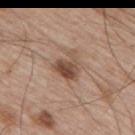Q: How was this image acquired?
A: ~15 mm tile from a whole-body skin photo
Q: What did automated image analysis measure?
A: a lesion area of about 7.5 mm² and a shape-asymmetry score of about 0.4 (0 = symmetric); border irregularity of about 4.5 on a 0–10 scale, internal color variation of about 5 on a 0–10 scale, and peripheral color asymmetry of about 1.5; a lesion-detection confidence of about 100/100
Q: What is the anatomic site?
A: the arm
Q: Patient demographics?
A: male, aged around 65
Q: What is the lesion's diameter?
A: ≈3.5 mm
Q: How was the tile lit?
A: white-light